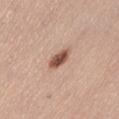Q: Is there a histopathology result?
A: imaged on a skin check; not biopsied
Q: What is the anatomic site?
A: the abdomen
Q: Who is the patient?
A: female, aged 48–52
Q: How was this image acquired?
A: ~15 mm tile from a whole-body skin photo